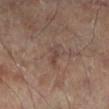Assessment:
Part of a total-body skin-imaging series; this lesion was reviewed on a skin check and was not flagged for biopsy.
Acquisition and patient details:
Imaged with cross-polarized lighting. The lesion is on the left lower leg. A male patient, aged approximately 65. Cropped from a total-body skin-imaging series; the visible field is about 15 mm.